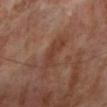Imaged during a routine full-body skin examination; the lesion was not biopsied and no histopathology is available.
The subject is a male approximately 70 years of age.
Cropped from a total-body skin-imaging series; the visible field is about 15 mm.
This is a cross-polarized tile.
The lesion is located on the left lower leg.
The lesion's longest dimension is about 4.5 mm.